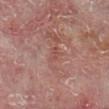<tbp_lesion>
<biopsy_status>not biopsied; imaged during a skin examination</biopsy_status>
<image>
  <source>total-body photography crop</source>
  <field_of_view_mm>15</field_of_view_mm>
</image>
<lighting>cross-polarized</lighting>
<lesion_size>
  <long_diameter_mm_approx>2.5</long_diameter_mm_approx>
</lesion_size>
<site>right lower leg</site>
<patient>
  <sex>male</sex>
  <age_approx>60</age_approx>
</patient>
</tbp_lesion>